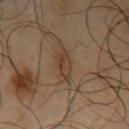Findings:
- follow-up · no biopsy performed (imaged during a skin exam)
- site · the left upper arm
- subject · male, approximately 65 years of age
- illumination · cross-polarized illumination
- size · ~3.5 mm (longest diameter)
- acquisition · ~15 mm crop, total-body skin-cancer survey